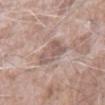The lesion was photographed on a routine skin check and not biopsied; there is no pathology result. A lesion tile, about 15 mm wide, cut from a 3D total-body photograph. A male patient, aged 73 to 77. On the right forearm. About 4 mm across. The tile uses white-light illumination. Automated tile analysis of the lesion measured border irregularity of about 3.5 on a 0–10 scale and a color-variation rating of about 4/10. The analysis additionally found an automated nevus-likeness rating near 0 out of 100 and a lesion-detection confidence of about 85/100.